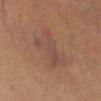Findings:
– notes · imaged on a skin check; not biopsied
– image · ~15 mm tile from a whole-body skin photo
– patient · female, in their mid-30s
– illumination · cross-polarized illumination
– automated metrics · an average lesion color of about L≈47 a*≈19 b*≈26 (CIELAB), a lesion–skin lightness drop of about 5, and a normalized lesion–skin contrast near 5.5; a classifier nevus-likeness of about 0/100
– anatomic site · the front of the torso